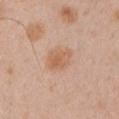workup: imaged on a skin check; not biopsied | acquisition: total-body-photography crop, ~15 mm field of view | lighting: white-light illumination | lesion diameter: ≈3.5 mm | patient: male, roughly 50 years of age | anatomic site: the arm.